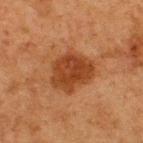body site: the upper back; imaging modality: ~15 mm tile from a whole-body skin photo; patient: male, aged around 75.